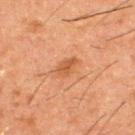Recorded during total-body skin imaging; not selected for excision or biopsy. Approximately 2.5 mm at its widest. The total-body-photography lesion software estimated a lesion color around L≈43 a*≈21 b*≈33 in CIELAB, a lesion–skin lightness drop of about 7, and a normalized lesion–skin contrast near 6.5. The lesion is located on the upper back. Cropped from a whole-body photographic skin survey; the tile spans about 15 mm. A male patient aged approximately 50. Imaged with cross-polarized lighting.